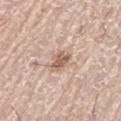Captured during whole-body skin photography for melanoma surveillance; the lesion was not biopsied.
The tile uses white-light illumination.
The recorded lesion diameter is about 3 mm.
A male patient, approximately 75 years of age.
A roughly 15 mm field-of-view crop from a total-body skin photograph.
The lesion is on the arm.